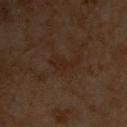Imaged during a routine full-body skin examination; the lesion was not biopsied and no histopathology is available.